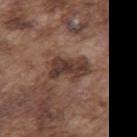A male patient, in their mid- to late 70s.
From the back.
The lesion's longest dimension is about 5 mm.
This is a white-light tile.
Automated tile analysis of the lesion measured a footprint of about 11 mm² and a symmetry-axis asymmetry near 0.3. It also reported a border-irregularity index near 3.5/10, a color-variation rating of about 4.5/10, and peripheral color asymmetry of about 1.5. The analysis additionally found a nevus-likeness score of about 5/100 and lesion-presence confidence of about 100/100.
Cropped from a whole-body photographic skin survey; the tile spans about 15 mm.